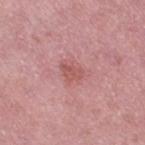Acquisition and patient details: A female subject, aged approximately 40. From the right thigh. A lesion tile, about 15 mm wide, cut from a 3D total-body photograph. The lesion's longest dimension is about 2.5 mm.